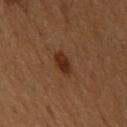Assessment: Part of a total-body skin-imaging series; this lesion was reviewed on a skin check and was not flagged for biopsy. Image and clinical context: On the upper back. Longest diameter approximately 2.5 mm. This is a cross-polarized tile. A female patient, about 50 years old. A 15 mm close-up tile from a total-body photography series done for melanoma screening.